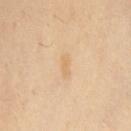Captured during whole-body skin photography for melanoma surveillance; the lesion was not biopsied. Located on the chest. A female subject approximately 40 years of age. The lesion-visualizer software estimated an area of roughly 2.5 mm², an eccentricity of roughly 0.9, and two-axis asymmetry of about 0.25. The analysis additionally found a border-irregularity index near 3/10, a color-variation rating of about 0/10, and a peripheral color-asymmetry measure near 0. The analysis additionally found a nevus-likeness score of about 0/100. A 15 mm crop from a total-body photograph taken for skin-cancer surveillance. The tile uses cross-polarized illumination.